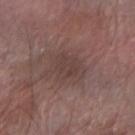Part of a total-body skin-imaging series; this lesion was reviewed on a skin check and was not flagged for biopsy. The total-body-photography lesion software estimated an average lesion color of about L≈39 a*≈17 b*≈19 (CIELAB), about 5 CIELAB-L* units darker than the surrounding skin, and a normalized border contrast of about 4.5. The analysis additionally found a border-irregularity index near 4/10 and peripheral color asymmetry of about 0. This is a white-light tile. About 3 mm across. Cropped from a total-body skin-imaging series; the visible field is about 15 mm. A male patient, in their mid-60s. On the right forearm.